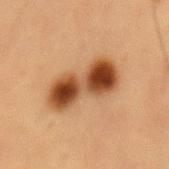subject: male, aged 53–57; lesion diameter: ~6.5 mm (longest diameter); body site: the abdomen; imaging modality: ~15 mm tile from a whole-body skin photo.